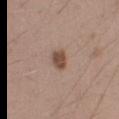Recorded during total-body skin imaging; not selected for excision or biopsy. This is a white-light tile. The lesion is on the right upper arm. The lesion-visualizer software estimated an outline eccentricity of about 0.55 (0 = round, 1 = elongated). The analysis additionally found border irregularity of about 3 on a 0–10 scale, a color-variation rating of about 2/10, and radial color variation of about 1. Cropped from a total-body skin-imaging series; the visible field is about 15 mm. A male subject aged around 20.